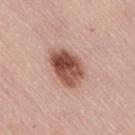The lesion is on the right thigh.
The subject is a female aged 63 to 67.
Cropped from a total-body skin-imaging series; the visible field is about 15 mm.